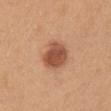Background:
About 4 mm across. A female patient, about 55 years old. A 15 mm close-up extracted from a 3D total-body photography capture. The lesion is located on the chest. The total-body-photography lesion software estimated a lesion area of about 11 mm² and a shape-asymmetry score of about 0.2 (0 = symmetric). And it measured a mean CIELAB color near L≈52 a*≈24 b*≈33, about 14 CIELAB-L* units darker than the surrounding skin, and a normalized lesion–skin contrast near 9.5. The analysis additionally found a border-irregularity rating of about 2/10, a within-lesion color-variation index near 5.5/10, and radial color variation of about 2. And it measured a classifier nevus-likeness of about 100/100 and a detector confidence of about 100 out of 100 that the crop contains a lesion.